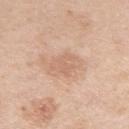The lesion was tiled from a total-body skin photograph and was not biopsied. A region of skin cropped from a whole-body photographic capture, roughly 15 mm wide. Imaged with white-light lighting. A female patient in their 40s. The lesion is located on the left upper arm. Automated image analysis of the tile measured a footprint of about 6.5 mm², an outline eccentricity of about 0.65 (0 = round, 1 = elongated), and two-axis asymmetry of about 0.35. The software also gave an average lesion color of about L≈65 a*≈20 b*≈31 (CIELAB), roughly 7 lightness units darker than nearby skin, and a lesion-to-skin contrast of about 4.5 (normalized; higher = more distinct). The analysis additionally found a nevus-likeness score of about 0/100 and a detector confidence of about 100 out of 100 that the crop contains a lesion.